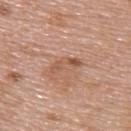workup = total-body-photography surveillance lesion; no biopsy
patient = female, in their 60s
image = total-body-photography crop, ~15 mm field of view
body site = the upper back
image-analysis metrics = roughly 8 lightness units darker than nearby skin and a normalized lesion–skin contrast near 6; a border-irregularity rating of about 4/10, a within-lesion color-variation index near 3.5/10, and peripheral color asymmetry of about 1; an automated nevus-likeness rating near 0 out of 100 and a detector confidence of about 100 out of 100 that the crop contains a lesion
diameter = ~4 mm (longest diameter)
illumination = white-light